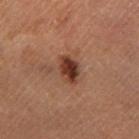follow-up: imaged on a skin check; not biopsied
diameter: ~3.5 mm (longest diameter)
patient: male, aged 48 to 52
imaging modality: ~15 mm tile from a whole-body skin photo
site: the left lower leg